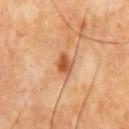Q: Is there a histopathology result?
A: catalogued during a skin exam; not biopsied
Q: What did automated image analysis measure?
A: a detector confidence of about 100 out of 100 that the crop contains a lesion
Q: What lighting was used for the tile?
A: cross-polarized illumination
Q: How was this image acquired?
A: total-body-photography crop, ~15 mm field of view
Q: Patient demographics?
A: male, aged around 65
Q: How large is the lesion?
A: ~2.5 mm (longest diameter)
Q: What is the anatomic site?
A: the chest